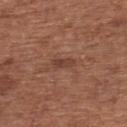  biopsy_status: not biopsied; imaged during a skin examination
  site: chest
  lesion_size:
    long_diameter_mm_approx: 3.0
  image:
    source: total-body photography crop
    field_of_view_mm: 15
  lighting: white-light
  patient:
    sex: male
    age_approx: 65